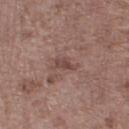Imaged during a routine full-body skin examination; the lesion was not biopsied and no histopathology is available. On the right lower leg. The subject is a male about 70 years old. The tile uses white-light illumination. Cropped from a whole-body photographic skin survey; the tile spans about 15 mm.